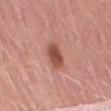Impression: Recorded during total-body skin imaging; not selected for excision or biopsy. Image and clinical context: A male patient aged approximately 80. The lesion's longest dimension is about 3.5 mm. The tile uses white-light illumination. From the left thigh. This image is a 15 mm lesion crop taken from a total-body photograph. Automated tile analysis of the lesion measured a footprint of about 7 mm², an eccentricity of roughly 0.8, and a shape-asymmetry score of about 0.2 (0 = symmetric). The analysis additionally found about 13 CIELAB-L* units darker than the surrounding skin and a lesion-to-skin contrast of about 8.5 (normalized; higher = more distinct).